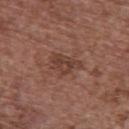This lesion was catalogued during total-body skin photography and was not selected for biopsy.
A close-up tile cropped from a whole-body skin photograph, about 15 mm across.
The patient is a female aged around 65.
Imaged with white-light lighting.
The lesion is located on the upper back.
The total-body-photography lesion software estimated a footprint of about 6 mm², a shape eccentricity near 0.8, and two-axis asymmetry of about 0.4. The software also gave a mean CIELAB color near L≈40 a*≈21 b*≈26, roughly 8 lightness units darker than nearby skin, and a normalized border contrast of about 6.5. And it measured a within-lesion color-variation index near 3/10. It also reported a nevus-likeness score of about 0/100 and a detector confidence of about 100 out of 100 that the crop contains a lesion.
Longest diameter approximately 4 mm.